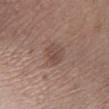The lesion was photographed on a routine skin check and not biopsied; there is no pathology result. Longest diameter approximately 2.5 mm. A male subject roughly 50 years of age. The lesion is located on the left lower leg. Captured under white-light illumination. A 15 mm close-up extracted from a 3D total-body photography capture.